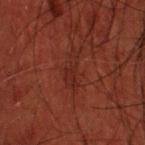Q: Is there a histopathology result?
A: catalogued during a skin exam; not biopsied
Q: What did automated image analysis measure?
A: roughly 4 lightness units darker than nearby skin and a lesion-to-skin contrast of about 5 (normalized; higher = more distinct); border irregularity of about 6 on a 0–10 scale, internal color variation of about 1.5 on a 0–10 scale, and radial color variation of about 0.5; an automated nevus-likeness rating near 25 out of 100
Q: Lesion location?
A: the head or neck
Q: How large is the lesion?
A: about 4 mm
Q: What is the imaging modality?
A: ~15 mm tile from a whole-body skin photo
Q: How was the tile lit?
A: cross-polarized illumination
Q: What are the patient's age and sex?
A: male, about 60 years old